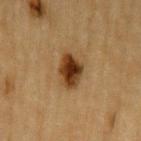Recorded during total-body skin imaging; not selected for excision or biopsy.
A roughly 15 mm field-of-view crop from a total-body skin photograph.
The lesion is located on the left upper arm.
A male subject in their mid-80s or older.
An algorithmic analysis of the crop reported border irregularity of about 2.5 on a 0–10 scale and internal color variation of about 5.5 on a 0–10 scale.
The recorded lesion diameter is about 4 mm.